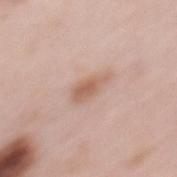Q: Was a biopsy performed?
A: imaged on a skin check; not biopsied
Q: What lighting was used for the tile?
A: white-light illumination
Q: What kind of image is this?
A: ~15 mm tile from a whole-body skin photo
Q: What is the lesion's diameter?
A: ~4 mm (longest diameter)
Q: What are the patient's age and sex?
A: female, aged 63–67
Q: Where on the body is the lesion?
A: the mid back